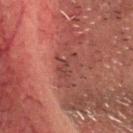{"biopsy_status": "not biopsied; imaged during a skin examination", "site": "head or neck", "automated_metrics": {"area_mm2_approx": 4.0, "eccentricity": 0.85, "shape_asymmetry": 0.55, "cielab_L": 31, "cielab_a": 21, "cielab_b": 20, "vs_skin_contrast_norm": 5.5, "border_irregularity_0_10": 7.0, "color_variation_0_10": 0.0, "peripheral_color_asymmetry": 0.0, "nevus_likeness_0_100": 0, "lesion_detection_confidence_0_100": 75}, "lesion_size": {"long_diameter_mm_approx": 3.0}, "lighting": "cross-polarized", "image": {"source": "total-body photography crop", "field_of_view_mm": 15}, "patient": {"sex": "male", "age_approx": 60}}